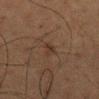Captured during whole-body skin photography for melanoma surveillance; the lesion was not biopsied. Captured under cross-polarized illumination. The patient is a male aged 58 to 62. This image is a 15 mm lesion crop taken from a total-body photograph. The recorded lesion diameter is about 3 mm. From the mid back.